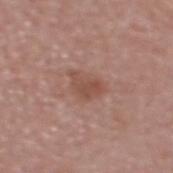Notes:
* follow-up: total-body-photography surveillance lesion; no biopsy
* subject: male, approximately 50 years of age
* automated lesion analysis: a lesion area of about 5.5 mm²; a classifier nevus-likeness of about 10/100
* image: ~15 mm crop, total-body skin-cancer survey
* body site: the head or neck
* lesion size: about 3.5 mm
* tile lighting: white-light